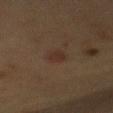Recorded during total-body skin imaging; not selected for excision or biopsy.